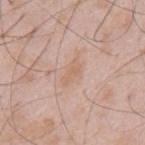Located on the mid back. The lesion's longest dimension is about 3 mm. Automated tile analysis of the lesion measured about 6 CIELAB-L* units darker than the surrounding skin. The software also gave border irregularity of about 4 on a 0–10 scale, internal color variation of about 1 on a 0–10 scale, and peripheral color asymmetry of about 0.5. The software also gave a nevus-likeness score of about 0/100. Cropped from a total-body skin-imaging series; the visible field is about 15 mm. A male patient about 50 years old.